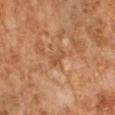This image is a 15 mm lesion crop taken from a total-body photograph. A male patient about 60 years old. Located on the right forearm.